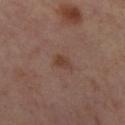Recorded during total-body skin imaging; not selected for excision or biopsy.
This is a cross-polarized tile.
Automated image analysis of the tile measured border irregularity of about 2.5 on a 0–10 scale.
Approximately 2.5 mm at its widest.
From the right lower leg.
A 15 mm close-up tile from a total-body photography series done for melanoma screening.
The subject is a female aged 53–57.